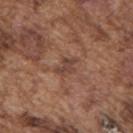This lesion was catalogued during total-body skin photography and was not selected for biopsy.
Imaged with white-light lighting.
A 15 mm close-up tile from a total-body photography series done for melanoma screening.
Automated tile analysis of the lesion measured a lesion area of about 4 mm², an eccentricity of roughly 0.75, and two-axis asymmetry of about 0.35. The software also gave an average lesion color of about L≈42 a*≈20 b*≈26 (CIELAB) and a normalized border contrast of about 6.5.
The lesion is located on the upper back.
A male patient aged around 75.